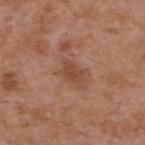* biopsy status — imaged on a skin check; not biopsied
* automated metrics — an area of roughly 4 mm² and a shape eccentricity near 0.75; a lesion color around L≈46 a*≈23 b*≈30 in CIELAB; a border-irregularity index near 3/10, a within-lesion color-variation index near 1.5/10, and radial color variation of about 0.5; a nevus-likeness score of about 0/100
* tile lighting — white-light
* patient — male, about 55 years old
* location — the upper back
* image — 15 mm crop, total-body photography